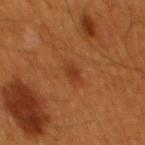Recorded during total-body skin imaging; not selected for excision or biopsy. A 15 mm close-up extracted from a 3D total-body photography capture. The patient is a male aged approximately 60. From the mid back. The recorded lesion diameter is about 3 mm. This is a cross-polarized tile.